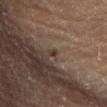Q: Was a biopsy performed?
A: imaged on a skin check; not biopsied
Q: Who is the patient?
A: male, roughly 60 years of age
Q: Lesion location?
A: the left lower leg
Q: Automated lesion metrics?
A: a lesion color around L≈30 a*≈15 b*≈20 in CIELAB, about 8 CIELAB-L* units darker than the surrounding skin, and a normalized border contrast of about 8
Q: What kind of image is this?
A: ~15 mm crop, total-body skin-cancer survey